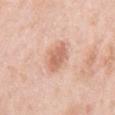workup = total-body-photography surveillance lesion; no biopsy
imaging modality = ~15 mm crop, total-body skin-cancer survey
lighting = white-light
size = ~3.5 mm (longest diameter)
site = the right upper arm
patient = female, aged approximately 65